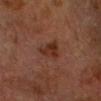{"biopsy_status": "not biopsied; imaged during a skin examination", "image": {"source": "total-body photography crop", "field_of_view_mm": 15}, "lesion_size": {"long_diameter_mm_approx": 3.0}, "automated_metrics": {"shape_asymmetry": 0.45, "cielab_L": 25, "cielab_a": 18, "cielab_b": 24, "vs_skin_darker_L": 7.0, "vs_skin_contrast_norm": 8.0, "nevus_likeness_0_100": 30}, "patient": {"sex": "male", "age_approx": 75}, "site": "head or neck", "lighting": "cross-polarized"}